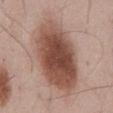Notes:
• notes — no biopsy performed (imaged during a skin exam)
• body site — the abdomen
• lesion diameter — ~9.5 mm (longest diameter)
• image-analysis metrics — a mean CIELAB color near L≈49 a*≈21 b*≈26 and roughly 16 lightness units darker than nearby skin; a border-irregularity rating of about 1.5/10, a within-lesion color-variation index near 6/10, and peripheral color asymmetry of about 2
• lighting — white-light illumination
• patient — male, in their mid- to late 50s
• acquisition — ~15 mm tile from a whole-body skin photo